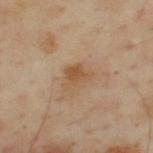<tbp_lesion>
<biopsy_status>not biopsied; imaged during a skin examination</biopsy_status>
<patient>
  <sex>male</sex>
  <age_approx>55</age_approx>
</patient>
<image>
  <source>total-body photography crop</source>
  <field_of_view_mm>15</field_of_view_mm>
</image>
<site>upper back</site>
</tbp_lesion>